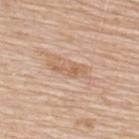The lesion was photographed on a routine skin check and not biopsied; there is no pathology result.
Located on the upper back.
Imaged with white-light lighting.
A male patient in their 80s.
A 15 mm close-up tile from a total-body photography series done for melanoma screening.